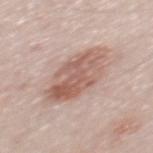  site: mid back
  lighting: white-light
  image:
    source: total-body photography crop
    field_of_view_mm: 15
  lesion_size:
    long_diameter_mm_approx: 7.0
  patient:
    sex: male
    age_approx: 50